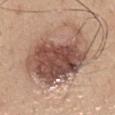The lesion is located on the mid back.
A male patient, roughly 35 years of age.
Measured at roughly 8.5 mm in maximum diameter.
Captured under white-light illumination.
A region of skin cropped from a whole-body photographic capture, roughly 15 mm wide.